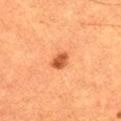Notes:
* follow-up: imaged on a skin check; not biopsied
* automated lesion analysis: a lesion area of about 4 mm² and two-axis asymmetry of about 0.3; a color-variation rating of about 2.5/10; a nevus-likeness score of about 95/100 and lesion-presence confidence of about 100/100
* acquisition: ~15 mm crop, total-body skin-cancer survey
* subject: male, approximately 60 years of age
* anatomic site: the left thigh
* size: about 2.5 mm